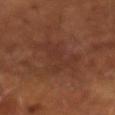Impression:
Imaged during a routine full-body skin examination; the lesion was not biopsied and no histopathology is available.
Context:
Cropped from a whole-body photographic skin survey; the tile spans about 15 mm. A male patient aged around 65. Longest diameter approximately 7 mm. Captured under cross-polarized illumination.